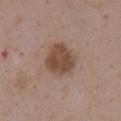The lesion is on the front of the torso.
A region of skin cropped from a whole-body photographic capture, roughly 15 mm wide.
A female patient, about 35 years old.